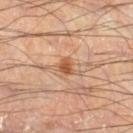Case summary:
- notes: total-body-photography surveillance lesion; no biopsy
- size: about 2 mm
- automated lesion analysis: an area of roughly 3 mm² and a symmetry-axis asymmetry near 0.35; a lesion–skin lightness drop of about 11; a border-irregularity rating of about 3/10, a color-variation rating of about 1.5/10, and peripheral color asymmetry of about 0.5
- acquisition: ~15 mm crop, total-body skin-cancer survey
- site: the right lower leg
- subject: male, aged 53–57
- illumination: cross-polarized